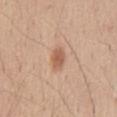Assessment: Part of a total-body skin-imaging series; this lesion was reviewed on a skin check and was not flagged for biopsy. Image and clinical context: Cropped from a total-body skin-imaging series; the visible field is about 15 mm. From the abdomen. A male patient, about 55 years old. Automated tile analysis of the lesion measured a lesion area of about 5 mm². The analysis additionally found roughly 10 lightness units darker than nearby skin and a normalized border contrast of about 7. And it measured a border-irregularity rating of about 2.5/10, internal color variation of about 2.5 on a 0–10 scale, and peripheral color asymmetry of about 0.5. It also reported an automated nevus-likeness rating near 95 out of 100. About 3.5 mm across. Captured under white-light illumination.